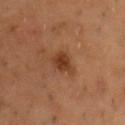Clinical impression: Recorded during total-body skin imaging; not selected for excision or biopsy. Background: Measured at roughly 3 mm in maximum diameter. A 15 mm crop from a total-body photograph taken for skin-cancer surveillance. Captured under cross-polarized illumination. From the chest. A male subject, aged 53–57.